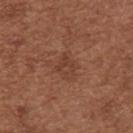<tbp_lesion>
  <biopsy_status>not biopsied; imaged during a skin examination</biopsy_status>
  <lighting>white-light</lighting>
  <image>
    <source>total-body photography crop</source>
    <field_of_view_mm>15</field_of_view_mm>
  </image>
  <automated_metrics>
    <eccentricity>0.7</eccentricity>
    <cielab_L>41</cielab_L>
    <cielab_a>22</cielab_a>
    <cielab_b>28</cielab_b>
    <vs_skin_contrast_norm>5.0</vs_skin_contrast_norm>
  </automated_metrics>
  <site>back</site>
  <patient>
    <sex>female</sex>
    <age_approx>40</age_approx>
  </patient>
  <lesion_size>
    <long_diameter_mm_approx>3.5</long_diameter_mm_approx>
  </lesion_size>
</tbp_lesion>